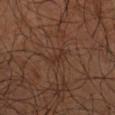Imaged during a routine full-body skin examination; the lesion was not biopsied and no histopathology is available. The subject is a male roughly 65 years of age. The lesion is on the left upper arm. Captured under cross-polarized illumination. This image is a 15 mm lesion crop taken from a total-body photograph. Longest diameter approximately 2.5 mm.